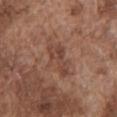Assessment:
Imaged during a routine full-body skin examination; the lesion was not biopsied and no histopathology is available.
Background:
From the chest. Captured under white-light illumination. A roughly 15 mm field-of-view crop from a total-body skin photograph. The subject is a male in their mid- to late 70s.